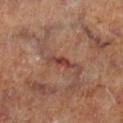This lesion was catalogued during total-body skin photography and was not selected for biopsy.
A 15 mm close-up extracted from a 3D total-body photography capture.
A male subject roughly 60 years of age.
Located on the right lower leg.
Automated image analysis of the tile measured a lesion area of about 2.5 mm², a shape eccentricity near 0.95, and two-axis asymmetry of about 0.25. The software also gave a lesion color around L≈39 a*≈24 b*≈25 in CIELAB and about 10 CIELAB-L* units darker than the surrounding skin. The software also gave a classifier nevus-likeness of about 0/100 and a detector confidence of about 65 out of 100 that the crop contains a lesion.
The lesion's longest dimension is about 3 mm.